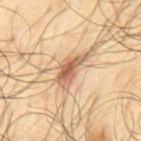image:
  source: total-body photography crop
  field_of_view_mm: 15
patient:
  sex: male
  age_approx: 65
site: back